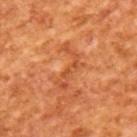Approximately 5.5 mm at its widest. A 15 mm crop from a total-body photograph taken for skin-cancer surveillance. This is a cross-polarized tile. A male subject aged approximately 65.